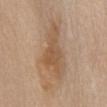Findings:
* biopsy status · catalogued during a skin exam; not biopsied
* location · the chest
* imaging modality · total-body-photography crop, ~15 mm field of view
* patient · female, approximately 65 years of age